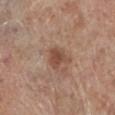Captured during whole-body skin photography for melanoma surveillance; the lesion was not biopsied. Located on the left lower leg. A male patient aged around 70. Captured under white-light illumination. Cropped from a whole-body photographic skin survey; the tile spans about 15 mm.